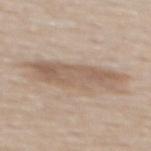Q: Was a biopsy performed?
A: total-body-photography surveillance lesion; no biopsy
Q: How was this image acquired?
A: 15 mm crop, total-body photography
Q: Patient demographics?
A: male, in their mid- to late 60s
Q: Automated lesion metrics?
A: a border-irregularity index near 4.5/10, a within-lesion color-variation index near 3.5/10, and radial color variation of about 1
Q: Lesion size?
A: ~9 mm (longest diameter)
Q: What is the anatomic site?
A: the mid back